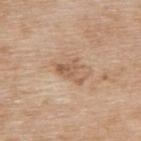Q: Is there a histopathology result?
A: catalogued during a skin exam; not biopsied
Q: What did automated image analysis measure?
A: a shape-asymmetry score of about 0.55 (0 = symmetric); an average lesion color of about L≈58 a*≈18 b*≈32 (CIELAB) and a lesion–skin lightness drop of about 9; a nevus-likeness score of about 0/100
Q: Patient demographics?
A: female, aged 73 to 77
Q: Where on the body is the lesion?
A: the upper back
Q: How was this image acquired?
A: ~15 mm crop, total-body skin-cancer survey